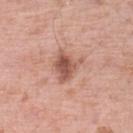The lesion was photographed on a routine skin check and not biopsied; there is no pathology result.